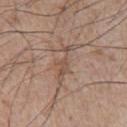No biopsy was performed on this lesion — it was imaged during a full skin examination and was not determined to be concerning.
This is a white-light tile.
The lesion's longest dimension is about 5.5 mm.
The total-body-photography lesion software estimated a lesion area of about 6.5 mm² and a shape-asymmetry score of about 0.5 (0 = symmetric). The software also gave a border-irregularity rating of about 8/10, internal color variation of about 3 on a 0–10 scale, and radial color variation of about 1. The analysis additionally found a nevus-likeness score of about 0/100 and a lesion-detection confidence of about 55/100.
On the chest.
A region of skin cropped from a whole-body photographic capture, roughly 15 mm wide.
The subject is a male in their mid- to late 70s.